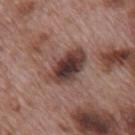Impression: Captured during whole-body skin photography for melanoma surveillance; the lesion was not biopsied. Clinical summary: A lesion tile, about 15 mm wide, cut from a 3D total-body photograph. The lesion's longest dimension is about 5.5 mm. The lesion is located on the mid back. An algorithmic analysis of the crop reported a lesion area of about 13 mm² and an eccentricity of roughly 0.8. The analysis additionally found a lesion–skin lightness drop of about 15 and a normalized border contrast of about 11.5. And it measured a border-irregularity rating of about 2.5/10. The analysis additionally found a nevus-likeness score of about 55/100 and lesion-presence confidence of about 100/100. This is a white-light tile. A male patient aged 68 to 72.